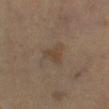{"biopsy_status": "not biopsied; imaged during a skin examination", "automated_metrics": {"nevus_likeness_0_100": 0, "lesion_detection_confidence_0_100": 100}, "patient": {"sex": "male", "age_approx": 65}, "image": {"source": "total-body photography crop", "field_of_view_mm": 15}, "lighting": "cross-polarized", "lesion_size": {"long_diameter_mm_approx": 3.5}}